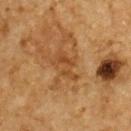biopsy status: total-body-photography surveillance lesion; no biopsy | automated lesion analysis: a nevus-likeness score of about 0/100 | acquisition: total-body-photography crop, ~15 mm field of view | anatomic site: the upper back | patient: male, aged around 85 or older | lighting: cross-polarized.